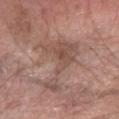Q: Was this lesion biopsied?
A: total-body-photography surveillance lesion; no biopsy
Q: What is the anatomic site?
A: the arm
Q: What lighting was used for the tile?
A: white-light illumination
Q: Patient demographics?
A: male, approximately 60 years of age
Q: What kind of image is this?
A: ~15 mm crop, total-body skin-cancer survey
Q: Lesion size?
A: ~7.5 mm (longest diameter)